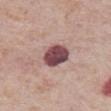notes: no biopsy performed (imaged during a skin exam) | illumination: white-light | lesion size: ~3.5 mm (longest diameter) | body site: the front of the torso | patient: male, in their mid- to late 70s | acquisition: 15 mm crop, total-body photography.